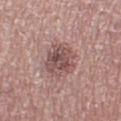{"patient": {"sex": "female", "age_approx": 40}, "lesion_size": {"long_diameter_mm_approx": 5.0}, "image": {"source": "total-body photography crop", "field_of_view_mm": 15}, "lighting": "white-light", "automated_metrics": {"eccentricity": 0.75, "shape_asymmetry": 0.15}, "site": "right thigh"}